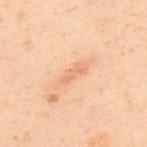{"biopsy_status": "not biopsied; imaged during a skin examination", "site": "upper back", "automated_metrics": {"area_mm2_approx": 4.0, "eccentricity": 0.9, "nevus_likeness_0_100": 0, "lesion_detection_confidence_0_100": 100}, "lighting": "cross-polarized", "patient": {"sex": "male", "age_approx": 50}, "lesion_size": {"long_diameter_mm_approx": 3.0}, "image": {"source": "total-body photography crop", "field_of_view_mm": 15}}